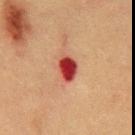Q: Is there a histopathology result?
A: no biopsy performed (imaged during a skin exam)
Q: What are the patient's age and sex?
A: male, aged around 50
Q: How was this image acquired?
A: 15 mm crop, total-body photography
Q: What did automated image analysis measure?
A: a lesion area of about 6.5 mm², an eccentricity of roughly 0.75, and a shape-asymmetry score of about 0.15 (0 = symmetric); a within-lesion color-variation index near 5.5/10 and a peripheral color-asymmetry measure near 2; an automated nevus-likeness rating near 0 out of 100
Q: What is the lesion's diameter?
A: about 3.5 mm
Q: How was the tile lit?
A: cross-polarized illumination
Q: What is the anatomic site?
A: the chest